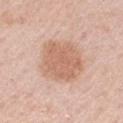No biopsy was performed on this lesion — it was imaged during a full skin examination and was not determined to be concerning. A male subject aged approximately 60. Measured at roughly 5 mm in maximum diameter. Imaged with white-light lighting. A close-up tile cropped from a whole-body skin photograph, about 15 mm across. The lesion is on the right upper arm.